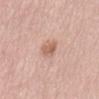Impression: Recorded during total-body skin imaging; not selected for excision or biopsy. Acquisition and patient details: Approximately 2.5 mm at its widest. The lesion is on the abdomen. The patient is a female about 65 years old. Cropped from a total-body skin-imaging series; the visible field is about 15 mm.